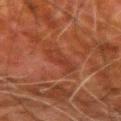No biopsy was performed on this lesion — it was imaged during a full skin examination and was not determined to be concerning. A 15 mm crop from a total-body photograph taken for skin-cancer surveillance. From the left upper arm. Approximately 3.5 mm at its widest. A male subject, in their 80s. The tile uses cross-polarized illumination. An algorithmic analysis of the crop reported a footprint of about 6 mm², an eccentricity of roughly 0.8, and a symmetry-axis asymmetry near 0.3. The analysis additionally found an average lesion color of about L≈30 a*≈23 b*≈27 (CIELAB) and about 5 CIELAB-L* units darker than the surrounding skin. The analysis additionally found a border-irregularity rating of about 3.5/10, a color-variation rating of about 2/10, and a peripheral color-asymmetry measure near 0.5. The software also gave an automated nevus-likeness rating near 0 out of 100 and a lesion-detection confidence of about 100/100.